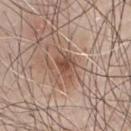The lesion was photographed on a routine skin check and not biopsied; there is no pathology result. A 15 mm close-up extracted from a 3D total-body photography capture. The total-body-photography lesion software estimated an area of roughly 6 mm², a shape eccentricity near 0.75, and a symmetry-axis asymmetry near 0.3. The software also gave a mean CIELAB color near L≈50 a*≈20 b*≈27, about 10 CIELAB-L* units darker than the surrounding skin, and a normalized border contrast of about 7.5. Approximately 3.5 mm at its widest. From the chest. The patient is a male aged approximately 80. This is a white-light tile.